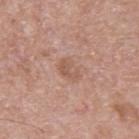No biopsy was performed on this lesion — it was imaged during a full skin examination and was not determined to be concerning. Automated image analysis of the tile measured a mean CIELAB color near L≈56 a*≈21 b*≈28 and about 7 CIELAB-L* units darker than the surrounding skin. And it measured a within-lesion color-variation index near 3/10 and radial color variation of about 1. It also reported an automated nevus-likeness rating near 0 out of 100. About 3 mm across. Cropped from a whole-body photographic skin survey; the tile spans about 15 mm. The tile uses white-light illumination. A male subject aged approximately 55.